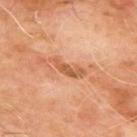Part of a total-body skin-imaging series; this lesion was reviewed on a skin check and was not flagged for biopsy. A 15 mm close-up tile from a total-body photography series done for melanoma screening. Longest diameter approximately 4.5 mm. A male subject in their mid-60s. On the upper back.